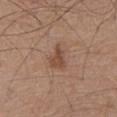{
  "biopsy_status": "not biopsied; imaged during a skin examination",
  "lighting": "white-light",
  "lesion_size": {
    "long_diameter_mm_approx": 3.0
  },
  "site": "abdomen",
  "image": {
    "source": "total-body photography crop",
    "field_of_view_mm": 15
  },
  "patient": {
    "sex": "male",
    "age_approx": 75
  },
  "automated_metrics": {
    "cielab_L": 47,
    "cielab_a": 20,
    "cielab_b": 29,
    "vs_skin_darker_L": 9.0,
    "vs_skin_contrast_norm": 7.0
  }
}